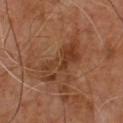| field | value |
|---|---|
| biopsy status | imaged on a skin check; not biopsied |
| imaging modality | 15 mm crop, total-body photography |
| automated lesion analysis | a mean CIELAB color near L≈37 a*≈22 b*≈31 and about 8 CIELAB-L* units darker than the surrounding skin; a border-irregularity rating of about 6.5/10, internal color variation of about 3.5 on a 0–10 scale, and radial color variation of about 1 |
| subject | male, aged 63–67 |